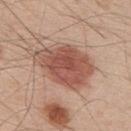Q: Was this lesion biopsied?
A: catalogued during a skin exam; not biopsied
Q: What are the patient's age and sex?
A: male, about 50 years old
Q: What is the imaging modality?
A: 15 mm crop, total-body photography
Q: How was the tile lit?
A: white-light
Q: Where on the body is the lesion?
A: the upper back
Q: What is the lesion's diameter?
A: ≈7.5 mm